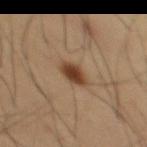notes = no biopsy performed (imaged during a skin exam); image source = total-body-photography crop, ~15 mm field of view; anatomic site = the front of the torso; subject = male, aged around 55; diameter = about 3 mm.